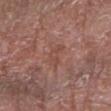Clinical impression: Captured during whole-body skin photography for melanoma surveillance; the lesion was not biopsied. Background: The total-body-photography lesion software estimated a lesion color around L≈47 a*≈23 b*≈25 in CIELAB. The software also gave border irregularity of about 5 on a 0–10 scale, internal color variation of about 1 on a 0–10 scale, and radial color variation of about 0.5. A close-up tile cropped from a whole-body skin photograph, about 15 mm across. Imaged with white-light lighting. The recorded lesion diameter is about 3.5 mm. The subject is a male aged approximately 80. Located on the right forearm.